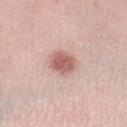illumination — white-light
imaging modality — ~15 mm tile from a whole-body skin photo
anatomic site — the leg
automated metrics — a mean CIELAB color near L≈60 a*≈23 b*≈24 and a lesion-to-skin contrast of about 8 (normalized; higher = more distinct)
lesion diameter — about 3.5 mm
subject — female, aged around 45